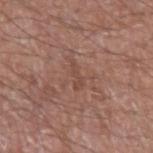Assessment: No biopsy was performed on this lesion — it was imaged during a full skin examination and was not determined to be concerning. Acquisition and patient details: On the left upper arm. Cropped from a total-body skin-imaging series; the visible field is about 15 mm. The patient is a male approximately 65 years of age.